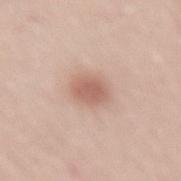biopsy_status: not biopsied; imaged during a skin examination
patient:
  sex: male
  age_approx: 65
lighting: white-light
image:
  source: total-body photography crop
  field_of_view_mm: 15
automated_metrics:
  area_mm2_approx: 6.0
  eccentricity: 0.55
  shape_asymmetry: 0.15
site: lower back